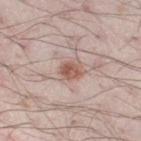This lesion was catalogued during total-body skin photography and was not selected for biopsy. A male patient aged around 25. From the right thigh. Approximately 2.5 mm at its widest. A 15 mm crop from a total-body photograph taken for skin-cancer surveillance. This is a white-light tile. An algorithmic analysis of the crop reported border irregularity of about 2.5 on a 0–10 scale, internal color variation of about 4 on a 0–10 scale, and radial color variation of about 1.5.